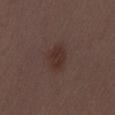The total-body-photography lesion software estimated a footprint of about 6 mm², a shape eccentricity near 0.7, and a symmetry-axis asymmetry near 0.2. The patient is a female aged 28 to 32. A close-up tile cropped from a whole-body skin photograph, about 15 mm across. The tile uses white-light illumination. From the lower back. The recorded lesion diameter is about 3.5 mm.